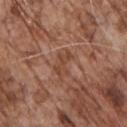A male subject in their 70s. Captured under white-light illumination. Cropped from a total-body skin-imaging series; the visible field is about 15 mm. Located on the chest. About 3 mm across.